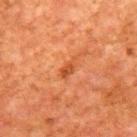The lesion was tiled from a total-body skin photograph and was not biopsied. Automated tile analysis of the lesion measured a footprint of about 3 mm², a shape eccentricity near 0.85, and two-axis asymmetry of about 0.35. The analysis additionally found lesion-presence confidence of about 100/100. The lesion is on the upper back. This image is a 15 mm lesion crop taken from a total-body photograph. A male subject aged approximately 80. Approximately 3 mm at its widest. This is a cross-polarized tile.